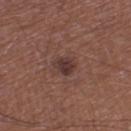The lesion is on the left thigh.
Measured at roughly 2.5 mm in maximum diameter.
A lesion tile, about 15 mm wide, cut from a 3D total-body photograph.
The tile uses white-light illumination.
The patient is a male approximately 65 years of age.